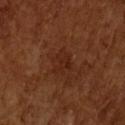Clinical impression: The lesion was photographed on a routine skin check and not biopsied; there is no pathology result. Acquisition and patient details: A male subject aged 63 to 67. About 3 mm across. The lesion-visualizer software estimated an area of roughly 4.5 mm², an eccentricity of roughly 0.6, and two-axis asymmetry of about 0.4. It also reported a border-irregularity rating of about 5/10, internal color variation of about 2 on a 0–10 scale, and radial color variation of about 0.5. This image is a 15 mm lesion crop taken from a total-body photograph. This is a cross-polarized tile.